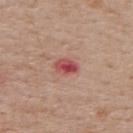Q: Was this lesion biopsied?
A: imaged on a skin check; not biopsied
Q: How was this image acquired?
A: ~15 mm tile from a whole-body skin photo
Q: How was the tile lit?
A: white-light illumination
Q: What is the lesion's diameter?
A: about 3 mm
Q: Lesion location?
A: the upper back
Q: What are the patient's age and sex?
A: male, approximately 70 years of age